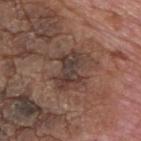The lesion was photographed on a routine skin check and not biopsied; there is no pathology result.
Captured under white-light illumination.
The lesion is located on the upper back.
A close-up tile cropped from a whole-body skin photograph, about 15 mm across.
Automated image analysis of the tile measured about 9 CIELAB-L* units darker than the surrounding skin and a normalized border contrast of about 8. The analysis additionally found a classifier nevus-likeness of about 0/100 and a detector confidence of about 100 out of 100 that the crop contains a lesion.
A male subject, about 75 years old.